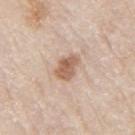{
  "biopsy_status": "not biopsied; imaged during a skin examination",
  "lighting": "white-light",
  "site": "right upper arm",
  "automated_metrics": {
    "cielab_L": 60,
    "cielab_a": 19,
    "cielab_b": 29,
    "vs_skin_darker_L": 12.0,
    "vs_skin_contrast_norm": 8.0,
    "nevus_likeness_0_100": 80
  },
  "image": {
    "source": "total-body photography crop",
    "field_of_view_mm": 15
  },
  "patient": {
    "sex": "male",
    "age_approx": 80
  },
  "lesion_size": {
    "long_diameter_mm_approx": 3.0
  }
}